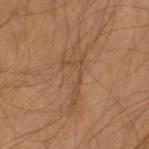follow-up = imaged on a skin check; not biopsied | site = the right upper arm | size = about 7 mm | imaging modality = ~15 mm crop, total-body skin-cancer survey | image-analysis metrics = a mean CIELAB color near L≈45 a*≈18 b*≈31 and a normalized lesion–skin contrast near 5; a classifier nevus-likeness of about 0/100 | lighting = cross-polarized illumination | patient = male, approximately 45 years of age.